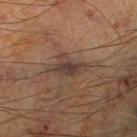This lesion was catalogued during total-body skin photography and was not selected for biopsy.
Longest diameter approximately 3 mm.
A male subject in their mid- to late 60s.
On the right thigh.
A roughly 15 mm field-of-view crop from a total-body skin photograph.